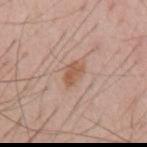biopsy_status: not biopsied; imaged during a skin examination
image:
  source: total-body photography crop
  field_of_view_mm: 15
site: mid back
lighting: white-light
lesion_size:
  long_diameter_mm_approx: 3.5
patient:
  sex: male
  age_approx: 55